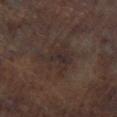Captured during whole-body skin photography for melanoma surveillance; the lesion was not biopsied. This is a cross-polarized tile. Located on the left lower leg. A 15 mm crop from a total-body photograph taken for skin-cancer surveillance. A male subject about 65 years old. Longest diameter approximately 5 mm.